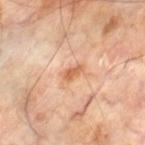Impression: No biopsy was performed on this lesion — it was imaged during a full skin examination and was not determined to be concerning. Context: A male subject, aged 68–72. Located on the left thigh. A region of skin cropped from a whole-body photographic capture, roughly 15 mm wide.